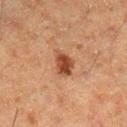Q: Is there a histopathology result?
A: catalogued during a skin exam; not biopsied
Q: Who is the patient?
A: female, aged around 50
Q: What kind of image is this?
A: 15 mm crop, total-body photography
Q: What lighting was used for the tile?
A: cross-polarized illumination
Q: Automated lesion metrics?
A: an area of roughly 6 mm², an outline eccentricity of about 0.7 (0 = round, 1 = elongated), and a shape-asymmetry score of about 0.3 (0 = symmetric); a border-irregularity index near 2.5/10, internal color variation of about 3.5 on a 0–10 scale, and peripheral color asymmetry of about 1.5; a classifier nevus-likeness of about 95/100 and a lesion-detection confidence of about 100/100
Q: Lesion location?
A: the right lower leg
Q: How large is the lesion?
A: ≈3 mm